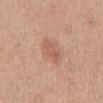Impression: This lesion was catalogued during total-body skin photography and was not selected for biopsy. Clinical summary: About 4 mm across. This is a white-light tile. A male patient, aged approximately 55. Cropped from a total-body skin-imaging series; the visible field is about 15 mm. The lesion is located on the mid back.